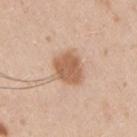No biopsy was performed on this lesion — it was imaged during a full skin examination and was not determined to be concerning.
A lesion tile, about 15 mm wide, cut from a 3D total-body photograph.
The tile uses white-light illumination.
Approximately 4 mm at its widest.
The lesion is on the right upper arm.
Automated tile analysis of the lesion measured a footprint of about 11 mm², an outline eccentricity of about 0.45 (0 = round, 1 = elongated), and a symmetry-axis asymmetry near 0.2. The software also gave a lesion color around L≈60 a*≈20 b*≈33 in CIELAB, a lesion–skin lightness drop of about 12, and a normalized border contrast of about 8. It also reported a classifier nevus-likeness of about 95/100 and a lesion-detection confidence of about 100/100.
The subject is a male about 35 years old.